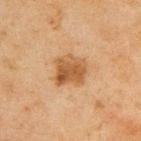The lesion was tiled from a total-body skin photograph and was not biopsied. Automated image analysis of the tile measured a mean CIELAB color near L≈45 a*≈19 b*≈34, about 10 CIELAB-L* units darker than the surrounding skin, and a normalized border contrast of about 8.5. A lesion tile, about 15 mm wide, cut from a 3D total-body photograph. Captured under cross-polarized illumination. A male patient, in their mid- to late 40s. On the back.